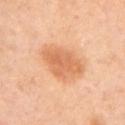Case summary:
* workup — imaged on a skin check; not biopsied
* image source — 15 mm crop, total-body photography
* site — the left upper arm
* subject — female, aged around 45